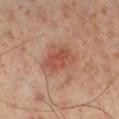follow-up = imaged on a skin check; not biopsied
anatomic site = the leg
diameter = ~5 mm (longest diameter)
subject = male, aged 53–57
image = 15 mm crop, total-body photography
tile lighting = cross-polarized illumination